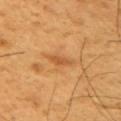notes — no biopsy performed (imaged during a skin exam)
location — the arm
automated lesion analysis — a lesion color around L≈55 a*≈25 b*≈44 in CIELAB and a lesion-to-skin contrast of about 6 (normalized; higher = more distinct); border irregularity of about 3 on a 0–10 scale, a within-lesion color-variation index near 1/10, and a peripheral color-asymmetry measure near 0
subject — male, aged 58–62
lesion diameter — about 2.5 mm
illumination — cross-polarized
imaging modality — 15 mm crop, total-body photography